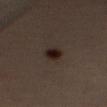Image and clinical context: A female subject roughly 45 years of age. A 15 mm crop from a total-body photograph taken for skin-cancer surveillance. The tile uses cross-polarized illumination. The lesion is located on the right upper arm. Automated image analysis of the tile measured an area of roughly 4.5 mm², an eccentricity of roughly 0.75, and two-axis asymmetry of about 0.25. The analysis additionally found an average lesion color of about L≈19 a*≈13 b*≈17 (CIELAB), roughly 10 lightness units darker than nearby skin, and a lesion-to-skin contrast of about 12 (normalized; higher = more distinct). The analysis additionally found a border-irregularity index near 2/10, a color-variation rating of about 4/10, and a peripheral color-asymmetry measure near 1.5. It also reported an automated nevus-likeness rating near 100 out of 100 and lesion-presence confidence of about 100/100.